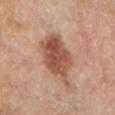follow-up: total-body-photography surveillance lesion; no biopsy | lighting: cross-polarized illumination | body site: the left lower leg | subject: male, approximately 65 years of age | image: total-body-photography crop, ~15 mm field of view | diameter: ~7 mm (longest diameter).